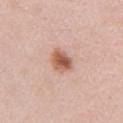Captured during whole-body skin photography for melanoma surveillance; the lesion was not biopsied. The recorded lesion diameter is about 3 mm. A female subject aged approximately 40. The lesion is on the left arm. This is a white-light tile. A 15 mm crop from a total-body photograph taken for skin-cancer surveillance. An algorithmic analysis of the crop reported a lesion color around L≈58 a*≈23 b*≈31 in CIELAB, roughly 14 lightness units darker than nearby skin, and a normalized border contrast of about 9.5. The analysis additionally found border irregularity of about 2 on a 0–10 scale, a color-variation rating of about 5/10, and peripheral color asymmetry of about 1.5. The software also gave a nevus-likeness score of about 100/100 and a detector confidence of about 100 out of 100 that the crop contains a lesion.